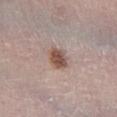Q: Was a biopsy performed?
A: imaged on a skin check; not biopsied
Q: How was this image acquired?
A: 15 mm crop, total-body photography
Q: Where on the body is the lesion?
A: the leg
Q: What is the lesion's diameter?
A: about 3 mm
Q: What are the patient's age and sex?
A: female, roughly 65 years of age
Q: Automated lesion metrics?
A: a lesion–skin lightness drop of about 13 and a lesion-to-skin contrast of about 9.5 (normalized; higher = more distinct); a border-irregularity index near 1.5/10, internal color variation of about 3.5 on a 0–10 scale, and radial color variation of about 1; a classifier nevus-likeness of about 95/100 and a detector confidence of about 100 out of 100 that the crop contains a lesion